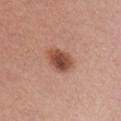workup = imaged on a skin check; not biopsied